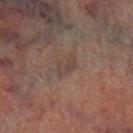Assessment: Part of a total-body skin-imaging series; this lesion was reviewed on a skin check and was not flagged for biopsy.